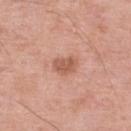biopsy status=no biopsy performed (imaged during a skin exam); location=the right lower leg; subject=male, approximately 80 years of age; imaging modality=15 mm crop, total-body photography.